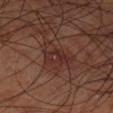biopsy status: catalogued during a skin exam; not biopsied | subject: male, aged around 70 | acquisition: 15 mm crop, total-body photography | anatomic site: the left lower leg | lighting: cross-polarized illumination | automated metrics: a footprint of about 5 mm²; an average lesion color of about L≈27 a*≈21 b*≈21 (CIELAB), about 5 CIELAB-L* units darker than the surrounding skin, and a lesion-to-skin contrast of about 6 (normalized; higher = more distinct) | size: about 3 mm.